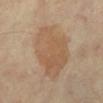biopsy status: total-body-photography surveillance lesion; no biopsy | subject: roughly 60 years of age | body site: the left lower leg | imaging modality: 15 mm crop, total-body photography.